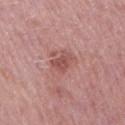This lesion was catalogued during total-body skin photography and was not selected for biopsy. A 15 mm close-up tile from a total-body photography series done for melanoma screening. The lesion is on the right upper arm. Captured under white-light illumination. An algorithmic analysis of the crop reported an area of roughly 4.5 mm² and an outline eccentricity of about 0.7 (0 = round, 1 = elongated). The analysis additionally found lesion-presence confidence of about 100/100. About 3 mm across. A female patient aged 48 to 52.